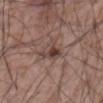{"biopsy_status": "not biopsied; imaged during a skin examination", "automated_metrics": {"area_mm2_approx": 6.0, "eccentricity": 0.65, "shape_asymmetry": 0.3, "cielab_L": 42, "cielab_a": 17, "cielab_b": 21, "vs_skin_darker_L": 9.0, "border_irregularity_0_10": 3.0, "color_variation_0_10": 8.0, "peripheral_color_asymmetry": 3.0}, "site": "abdomen", "patient": {"sex": "male", "age_approx": 60}, "image": {"source": "total-body photography crop", "field_of_view_mm": 15}}